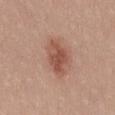– workup · total-body-photography surveillance lesion; no biopsy
– subject · female, aged approximately 20
– image-analysis metrics · a lesion area of about 10 mm², an outline eccentricity of about 0.8 (0 = round, 1 = elongated), and a shape-asymmetry score of about 0.2 (0 = symmetric); an average lesion color of about L≈52 a*≈23 b*≈28 (CIELAB), roughly 11 lightness units darker than nearby skin, and a normalized lesion–skin contrast near 7.5; a border-irregularity index near 2/10, internal color variation of about 4 on a 0–10 scale, and radial color variation of about 1.5
– body site · the back
– image source · ~15 mm tile from a whole-body skin photo
– tile lighting · white-light